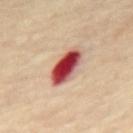Image and clinical context: Longest diameter approximately 5 mm. The lesion is located on the mid back. A male subject approximately 70 years of age. This image is a 15 mm lesion crop taken from a total-body photograph.